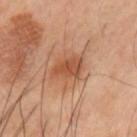follow-up — catalogued during a skin exam; not biopsied | tile lighting — cross-polarized illumination | lesion diameter — about 3 mm | subject — male, in their 60s | image-analysis metrics — a lesion color around L≈49 a*≈24 b*≈34 in CIELAB, roughly 10 lightness units darker than nearby skin, and a normalized border contrast of about 7.5; an automated nevus-likeness rating near 55 out of 100 and a lesion-detection confidence of about 100/100 | image source — ~15 mm tile from a whole-body skin photo | location — the arm.